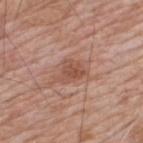workup — total-body-photography surveillance lesion; no biopsy
subject — male, aged 58 to 62
site — the upper back
tile lighting — white-light illumination
image — ~15 mm crop, total-body skin-cancer survey
lesion diameter — about 4 mm